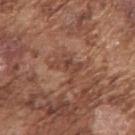Part of a total-body skin-imaging series; this lesion was reviewed on a skin check and was not flagged for biopsy. Approximately 4 mm at its widest. An algorithmic analysis of the crop reported an area of roughly 7 mm², a shape eccentricity near 0.65, and two-axis asymmetry of about 0.45. It also reported a lesion color around L≈44 a*≈22 b*≈28 in CIELAB, roughly 6 lightness units darker than nearby skin, and a lesion-to-skin contrast of about 5 (normalized; higher = more distinct). It also reported a nevus-likeness score of about 0/100 and a detector confidence of about 55 out of 100 that the crop contains a lesion. From the right upper arm. Imaged with white-light lighting. A male subject, aged around 75. A 15 mm crop from a total-body photograph taken for skin-cancer surveillance.